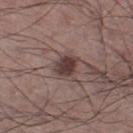Recorded during total-body skin imaging; not selected for excision or biopsy. An algorithmic analysis of the crop reported a footprint of about 5 mm², a shape eccentricity near 0.45, and a shape-asymmetry score of about 0.3 (0 = symmetric). It also reported a lesion color around L≈37 a*≈17 b*≈18 in CIELAB, about 13 CIELAB-L* units darker than the surrounding skin, and a normalized lesion–skin contrast near 11. It also reported border irregularity of about 2.5 on a 0–10 scale, a color-variation rating of about 4/10, and radial color variation of about 1.5. Imaged with white-light lighting. Located on the left thigh. A 15 mm close-up extracted from a 3D total-body photography capture. The lesion's longest dimension is about 2.5 mm. A male patient, in their mid- to late 50s.